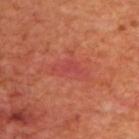No biopsy was performed on this lesion — it was imaged during a full skin examination and was not determined to be concerning. Approximately 4 mm at its widest. Located on the upper back. The tile uses cross-polarized illumination. A male patient aged around 70. A close-up tile cropped from a whole-body skin photograph, about 15 mm across.